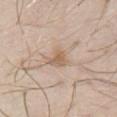Imaged during a routine full-body skin examination; the lesion was not biopsied and no histopathology is available.
Cropped from a total-body skin-imaging series; the visible field is about 15 mm.
This is a white-light tile.
An algorithmic analysis of the crop reported an outline eccentricity of about 0.65 (0 = round, 1 = elongated). The software also gave an average lesion color of about L≈61 a*≈15 b*≈30 (CIELAB), roughly 9 lightness units darker than nearby skin, and a lesion-to-skin contrast of about 6.5 (normalized; higher = more distinct). The analysis additionally found a border-irregularity rating of about 4/10, a within-lesion color-variation index near 2.5/10, and a peripheral color-asymmetry measure near 1.
The lesion is located on the chest.
Longest diameter approximately 3 mm.
A male patient, approximately 30 years of age.